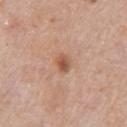Assessment: The lesion was tiled from a total-body skin photograph and was not biopsied. Clinical summary: A male patient about 80 years old. A close-up tile cropped from a whole-body skin photograph, about 15 mm across. Longest diameter approximately 2.5 mm. Located on the right upper arm.